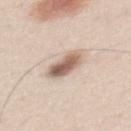follow-up — catalogued during a skin exam; not biopsied | subject — male, aged 23 to 27 | site — the mid back | imaging modality — 15 mm crop, total-body photography.